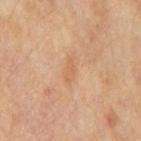Impression: Part of a total-body skin-imaging series; this lesion was reviewed on a skin check and was not flagged for biopsy. Acquisition and patient details: Approximately 3 mm at its widest. A male subject, aged 68 to 72. The lesion is on the mid back. This image is a 15 mm lesion crop taken from a total-body photograph.